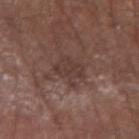Case summary:
* workup — imaged on a skin check; not biopsied
* subject — male, roughly 65 years of age
* image source — total-body-photography crop, ~15 mm field of view
* body site — the arm
* TBP lesion metrics — a classifier nevus-likeness of about 0/100 and a lesion-detection confidence of about 100/100